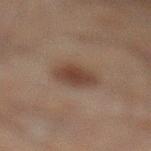lighting = cross-polarized illumination
diameter = ~3.5 mm (longest diameter)
imaging modality = ~15 mm tile from a whole-body skin photo
patient = male, approximately 60 years of age
automated metrics = a nevus-likeness score of about 100/100 and a lesion-detection confidence of about 100/100
location = the left lower leg